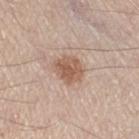<tbp_lesion>
  <biopsy_status>not biopsied; imaged during a skin examination</biopsy_status>
  <patient>
    <sex>male</sex>
    <age_approx>60</age_approx>
  </patient>
  <lighting>white-light</lighting>
  <image>
    <source>total-body photography crop</source>
    <field_of_view_mm>15</field_of_view_mm>
  </image>
  <site>right thigh</site>
  <automated_metrics>
    <eccentricity>0.6</eccentricity>
    <shape_asymmetry>0.2</shape_asymmetry>
    <border_irregularity_0_10>2.0</border_irregularity_0_10>
    <color_variation_0_10>3.0</color_variation_0_10>
    <peripheral_color_asymmetry>1.0</peripheral_color_asymmetry>
  </automated_metrics>
  <lesion_size>
    <long_diameter_mm_approx>3.5</long_diameter_mm_approx>
  </lesion_size>
</tbp_lesion>